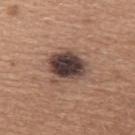Q: Was a biopsy performed?
A: no biopsy performed (imaged during a skin exam)
Q: What is the anatomic site?
A: the back
Q: Who is the patient?
A: female, in their mid- to late 50s
Q: How was this image acquired?
A: 15 mm crop, total-body photography